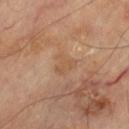  site: left thigh
  image:
    source: total-body photography crop
    field_of_view_mm: 15
  lesion_size:
    long_diameter_mm_approx: 3.0
  patient:
    sex: male
    age_approx: 70